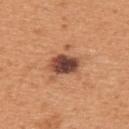  biopsy_status: not biopsied; imaged during a skin examination
  site: upper back
  lighting: white-light
  lesion_size:
    long_diameter_mm_approx: 4.0
  image:
    source: total-body photography crop
    field_of_view_mm: 15
  patient:
    sex: male
    age_approx: 55